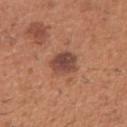biopsy_status: not biopsied; imaged during a skin examination
lighting: white-light
lesion_size:
  long_diameter_mm_approx: 3.0
site: right upper arm
automated_metrics:
  area_mm2_approx: 8.0
  eccentricity: 0.3
  shape_asymmetry: 0.15
  vs_skin_darker_L: 12.0
  vs_skin_contrast_norm: 9.0
  border_irregularity_0_10: 1.5
  color_variation_0_10: 4.0
  peripheral_color_asymmetry: 1.0
image:
  source: total-body photography crop
  field_of_view_mm: 15
patient:
  sex: male
  age_approx: 40